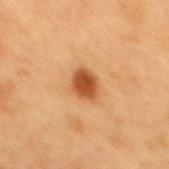{"biopsy_status": "not biopsied; imaged during a skin examination", "patient": {"sex": "female", "age_approx": 40}, "site": "mid back", "image": {"source": "total-body photography crop", "field_of_view_mm": 15}}